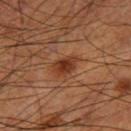Q: Was a biopsy performed?
A: catalogued during a skin exam; not biopsied
Q: Automated lesion metrics?
A: an area of roughly 5 mm², a shape eccentricity near 0.7, and two-axis asymmetry of about 0.25; a normalized lesion–skin contrast near 9; a border-irregularity rating of about 2.5/10 and peripheral color asymmetry of about 1
Q: What is the imaging modality?
A: ~15 mm crop, total-body skin-cancer survey
Q: What are the patient's age and sex?
A: male, about 60 years old
Q: How was the tile lit?
A: cross-polarized illumination
Q: Lesion location?
A: the right thigh